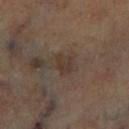follow-up: no biopsy performed (imaged during a skin exam)
image-analysis metrics: about 6 CIELAB-L* units darker than the surrounding skin and a lesion-to-skin contrast of about 6 (normalized; higher = more distinct); an automated nevus-likeness rating near 0 out of 100 and a detector confidence of about 100 out of 100 that the crop contains a lesion
location: the leg
tile lighting: cross-polarized
acquisition: ~15 mm tile from a whole-body skin photo